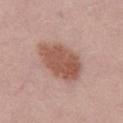Q: Was a biopsy performed?
A: total-body-photography surveillance lesion; no biopsy
Q: Patient demographics?
A: male, aged around 30
Q: How was this image acquired?
A: total-body-photography crop, ~15 mm field of view
Q: Illumination type?
A: white-light